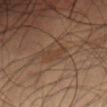Impression:
The lesion was photographed on a routine skin check and not biopsied; there is no pathology result.
Acquisition and patient details:
A male subject roughly 55 years of age. On the abdomen. This image is a 15 mm lesion crop taken from a total-body photograph.